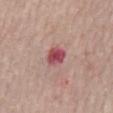Recorded during total-body skin imaging; not selected for excision or biopsy. The patient is a female approximately 65 years of age. The tile uses white-light illumination. From the mid back. This image is a 15 mm lesion crop taken from a total-body photograph. An algorithmic analysis of the crop reported a peripheral color-asymmetry measure near 1. The software also gave a classifier nevus-likeness of about 0/100 and a lesion-detection confidence of about 100/100. The lesion's longest dimension is about 2.5 mm.